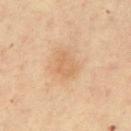* patient: female, aged 43 to 47
* body site: the abdomen
* image: ~15 mm tile from a whole-body skin photo
* TBP lesion metrics: a lesion area of about 6 mm², an eccentricity of roughly 0.8, and two-axis asymmetry of about 0.25